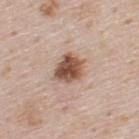Captured during whole-body skin photography for melanoma surveillance; the lesion was not biopsied.
A region of skin cropped from a whole-body photographic capture, roughly 15 mm wide.
Imaged with white-light lighting.
Automated tile analysis of the lesion measured a border-irregularity rating of about 2.5/10 and peripheral color asymmetry of about 1.5.
From the upper back.
The recorded lesion diameter is about 3.5 mm.
A male patient, about 45 years old.